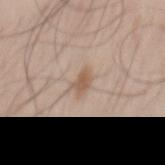The lesion is on the mid back. This is a white-light tile. About 2.5 mm across. A close-up tile cropped from a whole-body skin photograph, about 15 mm across. A male subject in their mid- to late 40s.